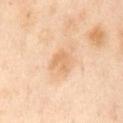No biopsy was performed on this lesion — it was imaged during a full skin examination and was not determined to be concerning. A roughly 15 mm field-of-view crop from a total-body skin photograph. The lesion-visualizer software estimated a lesion area of about 5.5 mm², a shape eccentricity near 0.6, and two-axis asymmetry of about 0.3. It also reported a lesion–skin lightness drop of about 7. It also reported a detector confidence of about 100 out of 100 that the crop contains a lesion. A female patient roughly 55 years of age. Longest diameter approximately 3 mm. Captured under cross-polarized illumination. On the abdomen.